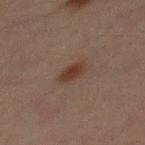follow-up: imaged on a skin check; not biopsied
automated lesion analysis: a border-irregularity rating of about 1.5/10, a within-lesion color-variation index near 2/10, and a peripheral color-asymmetry measure near 0.5
image: total-body-photography crop, ~15 mm field of view
lighting: cross-polarized illumination
lesion diameter: ≈2.5 mm
body site: the mid back
patient: male, about 30 years old